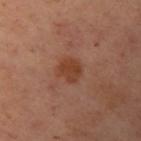Findings:
- follow-up · imaged on a skin check; not biopsied
- subject · female, in their mid-50s
- location · the left upper arm
- image · ~15 mm tile from a whole-body skin photo
- lighting · cross-polarized
- automated lesion analysis · an area of roughly 5.5 mm², a shape eccentricity near 0.55, and two-axis asymmetry of about 0.25; a lesion color around L≈33 a*≈20 b*≈26 in CIELAB, roughly 7 lightness units darker than nearby skin, and a lesion-to-skin contrast of about 7.5 (normalized; higher = more distinct); border irregularity of about 2 on a 0–10 scale and peripheral color asymmetry of about 0.5; an automated nevus-likeness rating near 35 out of 100 and a detector confidence of about 100 out of 100 that the crop contains a lesion